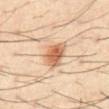Background: The lesion's longest dimension is about 4 mm. The subject is a male approximately 40 years of age. Located on the front of the torso. A 15 mm close-up extracted from a 3D total-body photography capture. This is a cross-polarized tile.